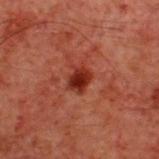Captured during whole-body skin photography for melanoma surveillance; the lesion was not biopsied. The subject is a male roughly 60 years of age. An algorithmic analysis of the crop reported roughly 11 lightness units darker than nearby skin. Longest diameter approximately 2.5 mm. Located on the back. The tile uses cross-polarized illumination. A 15 mm close-up extracted from a 3D total-body photography capture.